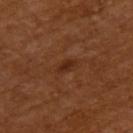Impression: Imaged during a routine full-body skin examination; the lesion was not biopsied and no histopathology is available. Acquisition and patient details: This is a cross-polarized tile. The lesion is located on the upper back. Automated tile analysis of the lesion measured a lesion color around L≈29 a*≈23 b*≈31 in CIELAB and about 7 CIELAB-L* units darker than the surrounding skin. The analysis additionally found an automated nevus-likeness rating near 45 out of 100 and a lesion-detection confidence of about 100/100. The lesion's longest dimension is about 2.5 mm. A close-up tile cropped from a whole-body skin photograph, about 15 mm across. A female subject in their mid-50s.